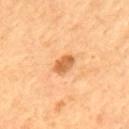Part of a total-body skin-imaging series; this lesion was reviewed on a skin check and was not flagged for biopsy. The lesion's longest dimension is about 3 mm. A male subject, roughly 55 years of age. Automated tile analysis of the lesion measured about 12 CIELAB-L* units darker than the surrounding skin and a lesion-to-skin contrast of about 8.5 (normalized; higher = more distinct). It also reported an automated nevus-likeness rating near 55 out of 100. This is a cross-polarized tile. A roughly 15 mm field-of-view crop from a total-body skin photograph. Located on the back.